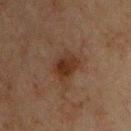site=the upper back | subject=male, roughly 65 years of age | lesion size=about 3.5 mm | automated lesion analysis=a footprint of about 7.5 mm², a shape eccentricity near 0.7, and a symmetry-axis asymmetry near 0.2; a border-irregularity index near 2/10 and a within-lesion color-variation index near 3.5/10 | image source=15 mm crop, total-body photography | illumination=cross-polarized illumination.